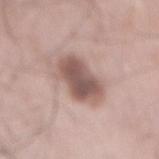Q: Is there a histopathology result?
A: catalogued during a skin exam; not biopsied
Q: What lighting was used for the tile?
A: white-light illumination
Q: Patient demographics?
A: male, aged 63 to 67
Q: Where on the body is the lesion?
A: the mid back
Q: How was this image acquired?
A: 15 mm crop, total-body photography